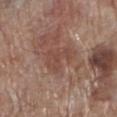* workup · catalogued during a skin exam; not biopsied
* patient · male, approximately 70 years of age
* illumination · white-light
* body site · the left lower leg
* image · 15 mm crop, total-body photography
* diameter · about 4 mm